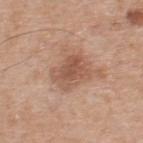The lesion was photographed on a routine skin check and not biopsied; there is no pathology result.
The lesion-visualizer software estimated a footprint of about 11 mm². The analysis additionally found a lesion-to-skin contrast of about 7 (normalized; higher = more distinct).
This is a white-light tile.
The patient is a male in their mid-50s.
Located on the back.
The recorded lesion diameter is about 4.5 mm.
A lesion tile, about 15 mm wide, cut from a 3D total-body photograph.